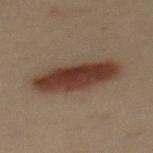Clinical impression:
Imaged during a routine full-body skin examination; the lesion was not biopsied and no histopathology is available.
Background:
Located on the back. This image is a 15 mm lesion crop taken from a total-body photograph. The patient is a female aged approximately 30.